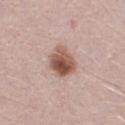Q: Was a biopsy performed?
A: catalogued during a skin exam; not biopsied
Q: What is the lesion's diameter?
A: ≈3.5 mm
Q: What did automated image analysis measure?
A: an area of roughly 9.5 mm², an eccentricity of roughly 0.5, and two-axis asymmetry of about 0.2; a lesion–skin lightness drop of about 15 and a normalized lesion–skin contrast near 10.5; a border-irregularity rating of about 2/10, a color-variation rating of about 7.5/10, and a peripheral color-asymmetry measure near 3
Q: Patient demographics?
A: male, about 30 years old
Q: What is the imaging modality?
A: total-body-photography crop, ~15 mm field of view
Q: Illumination type?
A: white-light illumination